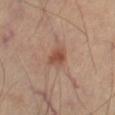<case>
  <biopsy_status>not biopsied; imaged during a skin examination</biopsy_status>
  <site>leg</site>
  <image>
    <source>total-body photography crop</source>
    <field_of_view_mm>15</field_of_view_mm>
  </image>
</case>